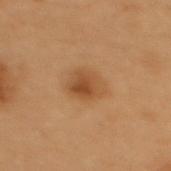Part of a total-body skin-imaging series; this lesion was reviewed on a skin check and was not flagged for biopsy.
Imaged with cross-polarized lighting.
The patient is a female aged 48–52.
Approximately 4 mm at its widest.
A region of skin cropped from a whole-body photographic capture, roughly 15 mm wide.
The lesion is on the upper back.
The lesion-visualizer software estimated a mean CIELAB color near L≈42 a*≈18 b*≈33 and about 8 CIELAB-L* units darker than the surrounding skin.